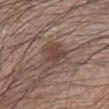Acquisition and patient details:
This is a white-light tile. Approximately 4 mm at its widest. The subject is a male in their mid-50s. A close-up tile cropped from a whole-body skin photograph, about 15 mm across. From the left forearm.